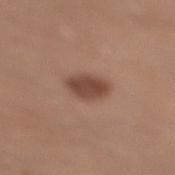Q: Was a biopsy performed?
A: catalogued during a skin exam; not biopsied
Q: What did automated image analysis measure?
A: an area of roughly 7.5 mm² and an eccentricity of roughly 0.7; roughly 12 lightness units darker than nearby skin; a detector confidence of about 100 out of 100 that the crop contains a lesion
Q: What are the patient's age and sex?
A: male, approximately 30 years of age
Q: How was the tile lit?
A: white-light
Q: What kind of image is this?
A: total-body-photography crop, ~15 mm field of view
Q: What is the anatomic site?
A: the leg